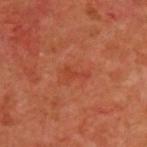Recorded during total-body skin imaging; not selected for excision or biopsy. Captured under cross-polarized illumination. A male patient about 60 years old. The lesion is located on the upper back. Approximately 3 mm at its widest. A region of skin cropped from a whole-body photographic capture, roughly 15 mm wide.